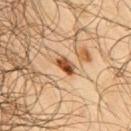workup = catalogued during a skin exam; not biopsied
automated lesion analysis = two-axis asymmetry of about 0.3; an average lesion color of about L≈48 a*≈24 b*≈36 (CIELAB) and a lesion–skin lightness drop of about 17; internal color variation of about 6 on a 0–10 scale and peripheral color asymmetry of about 2.5; a lesion-detection confidence of about 100/100
lighting = cross-polarized
site = the right upper arm
patient = male, in their mid- to late 60s
acquisition = ~15 mm crop, total-body skin-cancer survey
lesion diameter = ~2.5 mm (longest diameter)